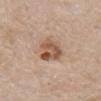| feature | finding |
|---|---|
| biopsy status | imaged on a skin check; not biopsied |
| location | the chest |
| TBP lesion metrics | a lesion color around L≈54 a*≈20 b*≈30 in CIELAB and a normalized border contrast of about 9; a border-irregularity index near 2.5/10, a within-lesion color-variation index near 6/10, and a peripheral color-asymmetry measure near 2; a classifier nevus-likeness of about 75/100 and a detector confidence of about 100 out of 100 that the crop contains a lesion |
| subject | male, approximately 60 years of age |
| lighting | white-light |
| image source | 15 mm crop, total-body photography |
| size | ≈4 mm |